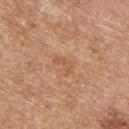Q: Is there a histopathology result?
A: no biopsy performed (imaged during a skin exam)